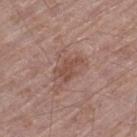No biopsy was performed on this lesion — it was imaged during a full skin examination and was not determined to be concerning. The recorded lesion diameter is about 3 mm. Imaged with white-light lighting. A 15 mm crop from a total-body photograph taken for skin-cancer surveillance. Located on the left thigh. The subject is a male aged around 70. The lesion-visualizer software estimated a classifier nevus-likeness of about 10/100 and lesion-presence confidence of about 100/100.